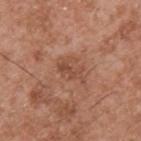The recorded lesion diameter is about 3 mm. Imaged with white-light lighting. From the upper back. A roughly 15 mm field-of-view crop from a total-body skin photograph. The patient is a male about 55 years old.